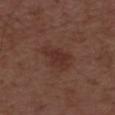Q: Was a biopsy performed?
A: catalogued during a skin exam; not biopsied
Q: What kind of image is this?
A: 15 mm crop, total-body photography
Q: Automated lesion metrics?
A: an average lesion color of about L≈32 a*≈20 b*≈23 (CIELAB) and a lesion-to-skin contrast of about 6.5 (normalized; higher = more distinct); an automated nevus-likeness rating near 40 out of 100 and a lesion-detection confidence of about 100/100
Q: Who is the patient?
A: male, aged 48 to 52
Q: Lesion size?
A: ≈4.5 mm